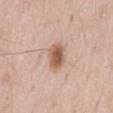<case>
<image>
  <source>total-body photography crop</source>
  <field_of_view_mm>15</field_of_view_mm>
</image>
<patient>
  <sex>male</sex>
  <age_approx>55</age_approx>
</patient>
<lesion_size>
  <long_diameter_mm_approx>3.0</long_diameter_mm_approx>
</lesion_size>
<lighting>white-light</lighting>
</case>